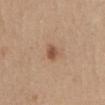Q: Was this lesion biopsied?
A: total-body-photography surveillance lesion; no biopsy
Q: What is the anatomic site?
A: the abdomen
Q: What lighting was used for the tile?
A: white-light
Q: Who is the patient?
A: female, about 40 years old
Q: Automated lesion metrics?
A: a border-irregularity index near 2.5/10, internal color variation of about 2 on a 0–10 scale, and radial color variation of about 0.5; a classifier nevus-likeness of about 80/100 and lesion-presence confidence of about 100/100
Q: What is the imaging modality?
A: total-body-photography crop, ~15 mm field of view
Q: What is the lesion's diameter?
A: about 2.5 mm